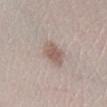<record>
  <biopsy_status>not biopsied; imaged during a skin examination</biopsy_status>
  <site>leg</site>
  <patient>
    <sex>female</sex>
    <age_approx>25</age_approx>
  </patient>
  <lesion_size>
    <long_diameter_mm_approx>3.5</long_diameter_mm_approx>
  </lesion_size>
  <lighting>white-light</lighting>
  <automated_metrics>
    <area_mm2_approx>6.5</area_mm2_approx>
    <eccentricity>0.7</eccentricity>
    <shape_asymmetry>0.1</shape_asymmetry>
    <border_irregularity_0_10>1.5</border_irregularity_0_10>
    <color_variation_0_10>2.0</color_variation_0_10>
    <peripheral_color_asymmetry>0.5</peripheral_color_asymmetry>
    <nevus_likeness_0_100>85</nevus_likeness_0_100>
    <lesion_detection_confidence_0_100>100</lesion_detection_confidence_0_100>
  </automated_metrics>
  <image>
    <source>total-body photography crop</source>
    <field_of_view_mm>15</field_of_view_mm>
  </image>
</record>